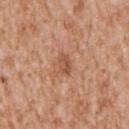| key | value |
|---|---|
| biopsy status | imaged on a skin check; not biopsied |
| location | the arm |
| size | about 2.5 mm |
| lighting | white-light |
| automated metrics | an automated nevus-likeness rating near 20 out of 100 and lesion-presence confidence of about 100/100 |
| patient | male, in their mid-40s |
| image | 15 mm crop, total-body photography |